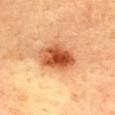Part of a total-body skin-imaging series; this lesion was reviewed on a skin check and was not flagged for biopsy. The tile uses cross-polarized illumination. A female patient, in their 60s. This image is a 15 mm lesion crop taken from a total-body photograph. The lesion is located on the mid back. Automated tile analysis of the lesion measured a mean CIELAB color near L≈50 a*≈27 b*≈38, a lesion–skin lightness drop of about 16, and a normalized border contrast of about 11.